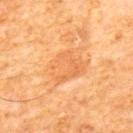Q: Was a biopsy performed?
A: total-body-photography surveillance lesion; no biopsy
Q: Who is the patient?
A: male, roughly 65 years of age
Q: What lighting was used for the tile?
A: cross-polarized
Q: How large is the lesion?
A: ~6 mm (longest diameter)
Q: Lesion location?
A: the mid back
Q: What kind of image is this?
A: ~15 mm crop, total-body skin-cancer survey